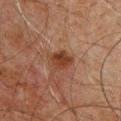Clinical impression: Part of a total-body skin-imaging series; this lesion was reviewed on a skin check and was not flagged for biopsy. Image and clinical context: Captured under cross-polarized illumination. A roughly 15 mm field-of-view crop from a total-body skin photograph. Automated image analysis of the tile measured a border-irregularity index near 2.5/10, a within-lesion color-variation index near 3.5/10, and peripheral color asymmetry of about 1. The software also gave a nevus-likeness score of about 60/100. On the chest. A male patient, approximately 60 years of age.